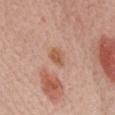Q: Was a biopsy performed?
A: no biopsy performed (imaged during a skin exam)
Q: What is the anatomic site?
A: the chest
Q: What is the lesion's diameter?
A: ~2.5 mm (longest diameter)
Q: Who is the patient?
A: female, aged 48 to 52
Q: What kind of image is this?
A: total-body-photography crop, ~15 mm field of view
Q: What lighting was used for the tile?
A: white-light illumination